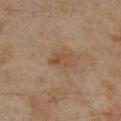On the right lower leg.
The subject is a female approximately 60 years of age.
About 2.5 mm across.
A region of skin cropped from a whole-body photographic capture, roughly 15 mm wide.